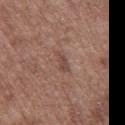Q: Lesion location?
A: the mid back
Q: What lighting was used for the tile?
A: white-light
Q: What is the imaging modality?
A: ~15 mm tile from a whole-body skin photo
Q: Lesion size?
A: about 3 mm
Q: What are the patient's age and sex?
A: male, aged 48 to 52